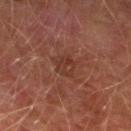Captured during whole-body skin photography for melanoma surveillance; the lesion was not biopsied. This is a cross-polarized tile. A male subject, aged approximately 75. A lesion tile, about 15 mm wide, cut from a 3D total-body photograph. The lesion is located on the left lower leg. Measured at roughly 3 mm in maximum diameter.